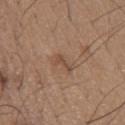Q: Was this lesion biopsied?
A: imaged on a skin check; not biopsied
Q: What is the anatomic site?
A: the chest
Q: Patient demographics?
A: male, about 65 years old
Q: What is the imaging modality?
A: total-body-photography crop, ~15 mm field of view